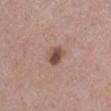This lesion was catalogued during total-body skin photography and was not selected for biopsy. A female patient, aged approximately 60. The tile uses white-light illumination. A 15 mm close-up tile from a total-body photography series done for melanoma screening. Located on the right lower leg.